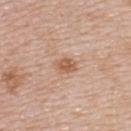Assessment:
Captured during whole-body skin photography for melanoma surveillance; the lesion was not biopsied.
Clinical summary:
Cropped from a whole-body photographic skin survey; the tile spans about 15 mm. On the upper back. A female subject, aged 43–47. The tile uses white-light illumination.